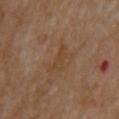This lesion was catalogued during total-body skin photography and was not selected for biopsy.
The subject is a female approximately 70 years of age.
From the upper back.
Cropped from a total-body skin-imaging series; the visible field is about 15 mm.
The tile uses cross-polarized illumination.
The recorded lesion diameter is about 4 mm.
The total-body-photography lesion software estimated an eccentricity of roughly 0.95 and a symmetry-axis asymmetry near 0.4. The software also gave border irregularity of about 5 on a 0–10 scale and a within-lesion color-variation index near 0/10. It also reported an automated nevus-likeness rating near 0 out of 100.